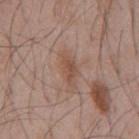patient: male, approximately 55 years of age
image: ~15 mm crop, total-body skin-cancer survey
automated lesion analysis: a border-irregularity index near 3.5/10, a color-variation rating of about 2.5/10, and peripheral color asymmetry of about 1; a classifier nevus-likeness of about 0/100
location: the chest
lighting: white-light
lesion diameter: about 3.5 mm